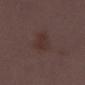  biopsy_status: not biopsied; imaged during a skin examination
  lesion_size:
    long_diameter_mm_approx: 4.0
  patient:
    sex: female
    age_approx: 30
  site: left thigh
  automated_metrics:
    cielab_L: 31
    cielab_a: 17
    cielab_b: 18
    vs_skin_darker_L: 5.0
    nevus_likeness_0_100: 15
    lesion_detection_confidence_0_100: 100
  image:
    source: total-body photography crop
    field_of_view_mm: 15